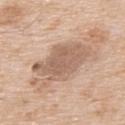workup: total-body-photography surveillance lesion; no biopsy | subject: male, about 65 years old | tile lighting: white-light illumination | image: total-body-photography crop, ~15 mm field of view | site: the back.